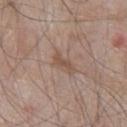| key | value |
|---|---|
| workup | no biopsy performed (imaged during a skin exam) |
| lesion size | about 3 mm |
| image-analysis metrics | an eccentricity of roughly 0.85 and two-axis asymmetry of about 0.35; a mean CIELAB color near L≈51 a*≈16 b*≈26, roughly 7 lightness units darker than nearby skin, and a lesion-to-skin contrast of about 6 (normalized; higher = more distinct); a border-irregularity rating of about 3.5/10 |
| anatomic site | the chest |
| tile lighting | white-light |
| acquisition | ~15 mm crop, total-body skin-cancer survey |
| subject | male, roughly 65 years of age |